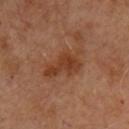This lesion was catalogued during total-body skin photography and was not selected for biopsy. A 15 mm crop from a total-body photograph taken for skin-cancer surveillance. Imaged with cross-polarized lighting. A male patient aged approximately 50. The recorded lesion diameter is about 5.5 mm. From the chest.